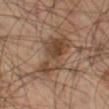biopsy status = total-body-photography surveillance lesion; no biopsy
image-analysis metrics = an eccentricity of roughly 0.85 and a symmetry-axis asymmetry near 0.4; a classifier nevus-likeness of about 55/100 and a lesion-detection confidence of about 100/100
diameter = about 6 mm
anatomic site = the left thigh
tile lighting = cross-polarized illumination
patient = male, in their mid-50s
image = 15 mm crop, total-body photography